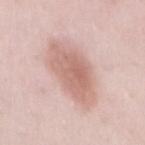- site — the back
- illumination — white-light illumination
- patient — female, aged 38–42
- image — ~15 mm crop, total-body skin-cancer survey